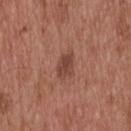Recorded during total-body skin imaging; not selected for excision or biopsy.
The patient is a male aged 53 to 57.
A 15 mm crop from a total-body photograph taken for skin-cancer surveillance.
Longest diameter approximately 3 mm.
The lesion-visualizer software estimated a classifier nevus-likeness of about 85/100 and a detector confidence of about 100 out of 100 that the crop contains a lesion.
Located on the head or neck.